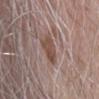workup: catalogued during a skin exam; not biopsied
patient: male, about 70 years old
site: the head or neck
illumination: white-light illumination
diameter: ~3.5 mm (longest diameter)
image source: 15 mm crop, total-body photography